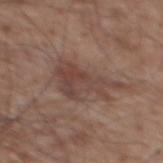The lesion was photographed on a routine skin check and not biopsied; there is no pathology result.
A 15 mm close-up tile from a total-body photography series done for melanoma screening.
An algorithmic analysis of the crop reported a lesion–skin lightness drop of about 8 and a normalized border contrast of about 6.5. And it measured a nevus-likeness score of about 0/100.
The lesion's longest dimension is about 6.5 mm.
The patient is a male in their mid- to late 50s.
From the mid back.
This is a white-light tile.